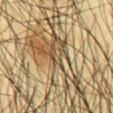Notes:
* subject · male, roughly 45 years of age
* imaging modality · total-body-photography crop, ~15 mm field of view
* automated lesion analysis · an area of roughly 21 mm², an eccentricity of roughly 0.95, and a shape-asymmetry score of about 0.6 (0 = symmetric); a border-irregularity index near 9.5/10, internal color variation of about 10 on a 0–10 scale, and radial color variation of about 3.5; an automated nevus-likeness rating near 0 out of 100 and lesion-presence confidence of about 0/100
* body site · the abdomen
* lesion diameter · ≈10.5 mm
* illumination · cross-polarized